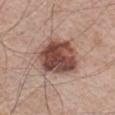<lesion>
<lighting>white-light</lighting>
<image>
  <source>total-body photography crop</source>
  <field_of_view_mm>15</field_of_view_mm>
</image>
<lesion_size>
  <long_diameter_mm_approx>5.5</long_diameter_mm_approx>
</lesion_size>
<patient>
  <sex>male</sex>
  <age_approx>65</age_approx>
</patient>
<site>front of the torso</site>
</lesion>